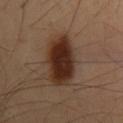Impression: Recorded during total-body skin imaging; not selected for excision or biopsy. Context: The lesion is on the mid back. About 7 mm across. A 15 mm close-up extracted from a 3D total-body photography capture. Imaged with cross-polarized lighting. A male subject roughly 55 years of age.